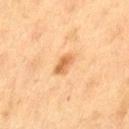<lesion>
  <biopsy_status>not biopsied; imaged during a skin examination</biopsy_status>
  <site>left thigh</site>
  <patient>
    <sex>female</sex>
    <age_approx>40</age_approx>
  </patient>
  <image>
    <source>total-body photography crop</source>
    <field_of_view_mm>15</field_of_view_mm>
  </image>
  <lesion_size>
    <long_diameter_mm_approx>3.0</long_diameter_mm_approx>
  </lesion_size>
</lesion>